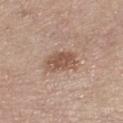  biopsy_status: not biopsied; imaged during a skin examination
  lighting: white-light
  patient:
    sex: female
    age_approx: 65
  image:
    source: total-body photography crop
    field_of_view_mm: 15
  site: right lower leg
  lesion_size:
    long_diameter_mm_approx: 4.0